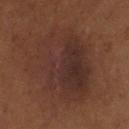Findings:
* lesion size — ≈8.5 mm
* lighting — cross-polarized illumination
* subject — female, about 80 years old
* imaging modality — total-body-photography crop, ~15 mm field of view
* automated lesion analysis — a border-irregularity rating of about 2.5/10, a color-variation rating of about 4.5/10, and radial color variation of about 1.5; a classifier nevus-likeness of about 5/100 and a lesion-detection confidence of about 100/100
* site — the right lower leg